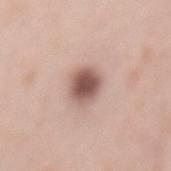notes = no biopsy performed (imaged during a skin exam) | subject = female, roughly 40 years of age | location = the back | lighting = white-light | imaging modality = ~15 mm tile from a whole-body skin photo.